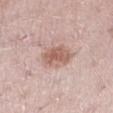| feature | finding |
|---|---|
| biopsy status | catalogued during a skin exam; not biopsied |
| patient | female, about 50 years old |
| automated lesion analysis | an area of roughly 9 mm², an eccentricity of roughly 0.7, and a symmetry-axis asymmetry near 0.15; a within-lesion color-variation index near 3.5/10 and peripheral color asymmetry of about 1; a classifier nevus-likeness of about 70/100 and lesion-presence confidence of about 100/100 |
| illumination | white-light |
| body site | the left lower leg |
| imaging modality | ~15 mm crop, total-body skin-cancer survey |
| lesion diameter | ≈4 mm |